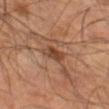The lesion was tiled from a total-body skin photograph and was not biopsied. A male patient, aged around 55. Automated image analysis of the tile measured a footprint of about 4 mm² and an outline eccentricity of about 0.8 (0 = round, 1 = elongated). And it measured a border-irregularity index near 2.5/10 and radial color variation of about 1. It also reported a classifier nevus-likeness of about 60/100 and a detector confidence of about 100 out of 100 that the crop contains a lesion. From the leg. A lesion tile, about 15 mm wide, cut from a 3D total-body photograph. The lesion's longest dimension is about 3 mm.